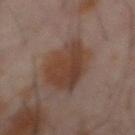Notes:
• image source: 15 mm crop, total-body photography
• subject: male, about 60 years old
• location: the abdomen